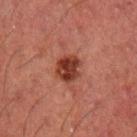Impression: The lesion was photographed on a routine skin check and not biopsied; there is no pathology result. Background: A male patient, approximately 50 years of age. On the left upper arm. The total-body-photography lesion software estimated an average lesion color of about L≈29 a*≈23 b*≈24 (CIELAB), roughly 11 lightness units darker than nearby skin, and a lesion-to-skin contrast of about 10.5 (normalized; higher = more distinct). And it measured a border-irregularity index near 2.5/10, a color-variation rating of about 4.5/10, and radial color variation of about 1.5. And it measured lesion-presence confidence of about 100/100. A lesion tile, about 15 mm wide, cut from a 3D total-body photograph.